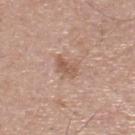Captured during whole-body skin photography for melanoma surveillance; the lesion was not biopsied.
A roughly 15 mm field-of-view crop from a total-body skin photograph.
Automated tile analysis of the lesion measured a lesion area of about 5.5 mm², an outline eccentricity of about 0.7 (0 = round, 1 = elongated), and a symmetry-axis asymmetry near 0.35. The software also gave internal color variation of about 2.5 on a 0–10 scale and peripheral color asymmetry of about 1. It also reported a nevus-likeness score of about 10/100.
The subject is a male roughly 55 years of age.
The tile uses white-light illumination.